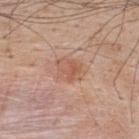Notes:
– biopsy status: catalogued during a skin exam; not biopsied
– subject: male, aged around 50
– image source: total-body-photography crop, ~15 mm field of view
– body site: the back